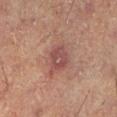biopsy status: imaged on a skin check; not biopsied | subject: male, roughly 50 years of age | diameter: about 4 mm | illumination: cross-polarized | image source: total-body-photography crop, ~15 mm field of view | anatomic site: the left lower leg | TBP lesion metrics: an outline eccentricity of about 0.6 (0 = round, 1 = elongated); a normalized lesion–skin contrast near 6.5.